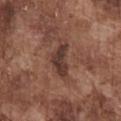A 15 mm crop from a total-body photograph taken for skin-cancer surveillance. The patient is a male aged 73 to 77. The lesion-visualizer software estimated a classifier nevus-likeness of about 0/100. From the chest.